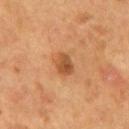Impression:
Captured during whole-body skin photography for melanoma surveillance; the lesion was not biopsied.
Background:
The patient is a male aged 53–57. A 15 mm close-up extracted from a 3D total-body photography capture. The lesion is located on the back.